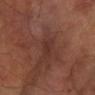The lesion was tiled from a total-body skin photograph and was not biopsied.